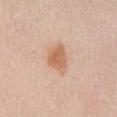image source=15 mm crop, total-body photography | patient=male, aged 53–57 | anatomic site=the abdomen | tile lighting=white-light | lesion diameter=~3.5 mm (longest diameter).